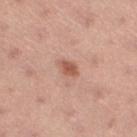follow-up: catalogued during a skin exam; not biopsied.